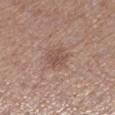The lesion was tiled from a total-body skin photograph and was not biopsied.
The recorded lesion diameter is about 3 mm.
A 15 mm crop from a total-body photograph taken for skin-cancer surveillance.
This is a white-light tile.
A female subject aged 38 to 42.
The lesion is located on the leg.